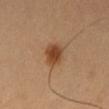The lesion was tiled from a total-body skin photograph and was not biopsied.
The lesion is on the left thigh.
Approximately 3.5 mm at its widest.
Cropped from a total-body skin-imaging series; the visible field is about 15 mm.
A male patient about 50 years old.